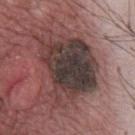The lesion was tiled from a total-body skin photograph and was not biopsied. A male patient aged approximately 65. The total-body-photography lesion software estimated a shape eccentricity near 0.6 and two-axis asymmetry of about 0.3. The software also gave border irregularity of about 5 on a 0–10 scale, a color-variation rating of about 8/10, and a peripheral color-asymmetry measure near 2.5. The analysis additionally found a classifier nevus-likeness of about 0/100. A 15 mm close-up tile from a total-body photography series done for melanoma screening. Approximately 8.5 mm at its widest. On the chest. The tile uses white-light illumination.